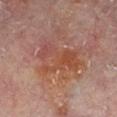On the left lower leg. Approximately 5.5 mm at its widest. The tile uses cross-polarized illumination. A male patient roughly 60 years of age. Cropped from a whole-body photographic skin survey; the tile spans about 15 mm.